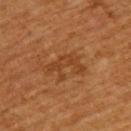Case summary:
* follow-up · imaged on a skin check; not biopsied
* automated metrics · an area of roughly 6.5 mm², an eccentricity of roughly 0.8, and a shape-asymmetry score of about 0.7 (0 = symmetric)
* lesion size · ≈4 mm
* site · the upper back
* lighting · cross-polarized illumination
* image · 15 mm crop, total-body photography
* patient · male, in their 60s